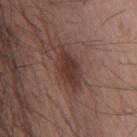Part of a total-body skin-imaging series; this lesion was reviewed on a skin check and was not flagged for biopsy.